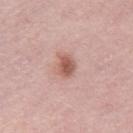follow-up: catalogued during a skin exam; not biopsied
automated lesion analysis: an area of roughly 5 mm², a shape eccentricity near 0.65, and two-axis asymmetry of about 0.15; a mean CIELAB color near L≈57 a*≈23 b*≈26 and a lesion–skin lightness drop of about 12; a within-lesion color-variation index near 3/10 and peripheral color asymmetry of about 1; an automated nevus-likeness rating near 75 out of 100
image source: ~15 mm tile from a whole-body skin photo
anatomic site: the right thigh
lesion diameter: ≈2.5 mm
illumination: white-light
patient: female, aged around 65